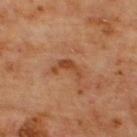<case>
<biopsy_status>not biopsied; imaged during a skin examination</biopsy_status>
<image>
  <source>total-body photography crop</source>
  <field_of_view_mm>15</field_of_view_mm>
</image>
<site>back</site>
<patient>
  <sex>male</sex>
  <age_approx>65</age_approx>
</patient>
</case>